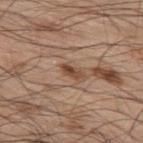Assessment: Recorded during total-body skin imaging; not selected for excision or biopsy. Context: A male subject, about 70 years old. Located on the back. Imaged with white-light lighting. A 15 mm close-up extracted from a 3D total-body photography capture.